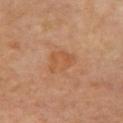| feature | finding |
|---|---|
| notes | no biopsy performed (imaged during a skin exam) |
| location | the chest |
| imaging modality | total-body-photography crop, ~15 mm field of view |
| patient | female, roughly 65 years of age |
| lighting | cross-polarized illumination |